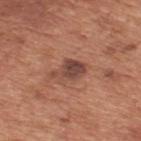Notes:
– follow-up · total-body-photography surveillance lesion; no biopsy
– lighting · white-light
– image-analysis metrics · an eccentricity of roughly 0.85 and a shape-asymmetry score of about 0.35 (0 = symmetric); border irregularity of about 4 on a 0–10 scale, a within-lesion color-variation index near 3.5/10, and radial color variation of about 1
– image source · total-body-photography crop, ~15 mm field of view
– lesion diameter · ~4 mm (longest diameter)
– site · the upper back
– patient · male, in their mid- to late 60s